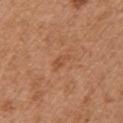Recorded during total-body skin imaging; not selected for excision or biopsy. Cropped from a whole-body photographic skin survey; the tile spans about 15 mm. From the chest. About 2.5 mm across. Imaged with white-light lighting. A female subject approximately 40 years of age.